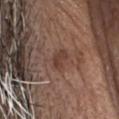Part of a total-body skin-imaging series; this lesion was reviewed on a skin check and was not flagged for biopsy. Measured at roughly 2.5 mm in maximum diameter. A male patient aged 73–77. An algorithmic analysis of the crop reported a footprint of about 4 mm², an outline eccentricity of about 0.8 (0 = round, 1 = elongated), and a symmetry-axis asymmetry near 0.35. The analysis additionally found border irregularity of about 3.5 on a 0–10 scale, internal color variation of about 2 on a 0–10 scale, and radial color variation of about 0.5. It also reported a nevus-likeness score of about 0/100 and lesion-presence confidence of about 100/100. The lesion is on the head or neck. The tile uses white-light illumination. This image is a 15 mm lesion crop taken from a total-body photograph.